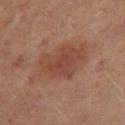Impression: Imaged during a routine full-body skin examination; the lesion was not biopsied and no histopathology is available. Image and clinical context: A female patient, aged 48 to 52. The recorded lesion diameter is about 5 mm. This image is a 15 mm lesion crop taken from a total-body photograph. The tile uses cross-polarized illumination. An algorithmic analysis of the crop reported an area of roughly 16 mm², an eccentricity of roughly 0.6, and two-axis asymmetry of about 0.25. The analysis additionally found a border-irregularity rating of about 3/10, a color-variation rating of about 3/10, and a peripheral color-asymmetry measure near 1. It also reported a nevus-likeness score of about 80/100 and lesion-presence confidence of about 100/100. On the left thigh.